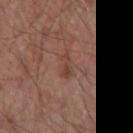An algorithmic analysis of the crop reported a normalized border contrast of about 6. On the right forearm. Imaged with white-light lighting. A male subject, in their mid-50s. A 15 mm close-up extracted from a 3D total-body photography capture.